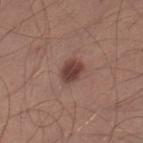{
  "biopsy_status": "not biopsied; imaged during a skin examination",
  "image": {
    "source": "total-body photography crop",
    "field_of_view_mm": 15
  },
  "patient": {
    "sex": "male",
    "age_approx": 30
  },
  "lesion_size": {
    "long_diameter_mm_approx": 2.5
  },
  "lighting": "white-light",
  "site": "right lower leg",
  "automated_metrics": {
    "eccentricity": 0.5,
    "cielab_L": 41,
    "cielab_a": 20,
    "cielab_b": 23,
    "vs_skin_darker_L": 12.0
  }
}